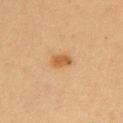<record>
<biopsy_status>not biopsied; imaged during a skin examination</biopsy_status>
<patient>
  <sex>female</sex>
  <age_approx>40</age_approx>
</patient>
<lighting>cross-polarized</lighting>
<site>left upper arm</site>
<lesion_size>
  <long_diameter_mm_approx>3.0</long_diameter_mm_approx>
</lesion_size>
<image>
  <source>total-body photography crop</source>
  <field_of_view_mm>15</field_of_view_mm>
</image>
<automated_metrics>
  <area_mm2_approx>4.5</area_mm2_approx>
  <eccentricity>0.75</eccentricity>
  <shape_asymmetry>0.2</shape_asymmetry>
  <border_irregularity_0_10>1.5</border_irregularity_0_10>
  <color_variation_0_10>2.0</color_variation_0_10>
  <nevus_likeness_0_100>90</nevus_likeness_0_100>
  <lesion_detection_confidence_0_100>100</lesion_detection_confidence_0_100>
</automated_metrics>
</record>